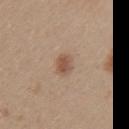  biopsy_status: not biopsied; imaged during a skin examination
  lighting: white-light
  image:
    source: total-body photography crop
    field_of_view_mm: 15
  site: left upper arm
  patient:
    sex: female
    age_approx: 25
  automated_metrics:
    area_mm2_approx: 4.5
    eccentricity: 0.5
    shape_asymmetry: 0.1
    cielab_L: 53
    cielab_a: 18
    cielab_b: 30
    vs_skin_darker_L: 10.0
    vs_skin_contrast_norm: 7.0
    border_irregularity_0_10: 1.0
    peripheral_color_asymmetry: 1.0
    nevus_likeness_0_100: 90
    lesion_detection_confidence_0_100: 100